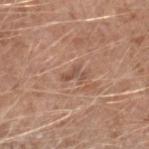follow-up: no biopsy performed (imaged during a skin exam) | body site: the arm | diameter: about 2.5 mm | patient: male, aged approximately 60 | image source: ~15 mm crop, total-body skin-cancer survey | automated lesion analysis: an area of roughly 3 mm², a shape eccentricity near 0.85, and two-axis asymmetry of about 0.55; an average lesion color of about L≈52 a*≈20 b*≈27 (CIELAB), roughly 8 lightness units darker than nearby skin, and a lesion-to-skin contrast of about 6 (normalized; higher = more distinct); a border-irregularity index near 6/10, a within-lesion color-variation index near 0/10, and radial color variation of about 0; a nevus-likeness score of about 0/100 and a detector confidence of about 95 out of 100 that the crop contains a lesion | lighting: white-light illumination.